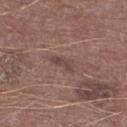<case>
<biopsy_status>not biopsied; imaged during a skin examination</biopsy_status>
<patient>
  <sex>male</sex>
  <age_approx>75</age_approx>
</patient>
<image>
  <source>total-body photography crop</source>
  <field_of_view_mm>15</field_of_view_mm>
</image>
<site>right lower leg</site>
<lesion_size>
  <long_diameter_mm_approx>3.0</long_diameter_mm_approx>
</lesion_size>
<lighting>white-light</lighting>
</case>